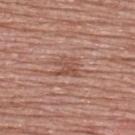location: the upper back
image: total-body-photography crop, ~15 mm field of view
automated lesion analysis: an eccentricity of roughly 0.6; a lesion color around L≈51 a*≈22 b*≈28 in CIELAB, roughly 8 lightness units darker than nearby skin, and a lesion-to-skin contrast of about 6 (normalized; higher = more distinct); a classifier nevus-likeness of about 0/100 and a detector confidence of about 70 out of 100 that the crop contains a lesion
tile lighting: white-light
lesion diameter: about 3 mm
subject: male, aged around 50